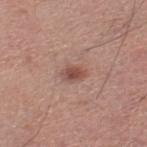No biopsy was performed on this lesion — it was imaged during a full skin examination and was not determined to be concerning. Cropped from a total-body skin-imaging series; the visible field is about 15 mm. The lesion is on the right lower leg. A male subject aged around 60.